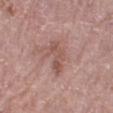Acquisition and patient details: The patient is a female roughly 60 years of age. Located on the right lower leg. A 15 mm close-up extracted from a 3D total-body photography capture.